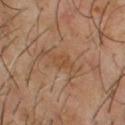follow-up = total-body-photography surveillance lesion; no biopsy | illumination = cross-polarized | site = the upper back | imaging modality = 15 mm crop, total-body photography | TBP lesion metrics = a footprint of about 3 mm² and a symmetry-axis asymmetry near 0.35; border irregularity of about 3.5 on a 0–10 scale, a color-variation rating of about 2/10, and a peripheral color-asymmetry measure near 0.5 | patient = male, in their mid-60s | size = ~2.5 mm (longest diameter).